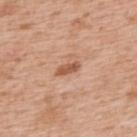This lesion was catalogued during total-body skin photography and was not selected for biopsy. About 3 mm across. A male subject aged 63–67. This is a white-light tile. A roughly 15 mm field-of-view crop from a total-body skin photograph. The lesion is located on the back.